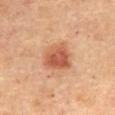Clinical impression: No biopsy was performed on this lesion — it was imaged during a full skin examination and was not determined to be concerning. Acquisition and patient details: Imaged with cross-polarized lighting. The lesion is on the chest. The recorded lesion diameter is about 4 mm. A female subject, in their 60s. A roughly 15 mm field-of-view crop from a total-body skin photograph.